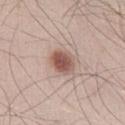Findings:
• biopsy status · catalogued during a skin exam; not biopsied
• TBP lesion metrics · a symmetry-axis asymmetry near 0.15; a mean CIELAB color near L≈55 a*≈20 b*≈24, roughly 14 lightness units darker than nearby skin, and a lesion-to-skin contrast of about 9 (normalized; higher = more distinct); internal color variation of about 4.5 on a 0–10 scale and peripheral color asymmetry of about 1.5
• image · total-body-photography crop, ~15 mm field of view
• location · the right thigh
• lesion size · about 3 mm
• patient · male, aged 23 to 27